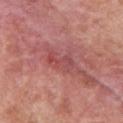Q: Is there a histopathology result?
A: catalogued during a skin exam; not biopsied
Q: What kind of image is this?
A: 15 mm crop, total-body photography
Q: How was the tile lit?
A: white-light
Q: Who is the patient?
A: female, roughly 50 years of age
Q: Lesion location?
A: the front of the torso
Q: Lesion size?
A: about 3.5 mm